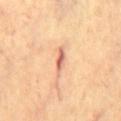The recorded lesion diameter is about 4 mm. On the chest. Captured under cross-polarized illumination. A male subject aged 68–72. A 15 mm close-up tile from a total-body photography series done for melanoma screening.